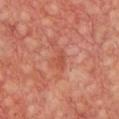The lesion was photographed on a routine skin check and not biopsied; there is no pathology result. The patient is a female aged 43 to 47. An algorithmic analysis of the crop reported an area of roughly 4.5 mm² and an outline eccentricity of about 0.75 (0 = round, 1 = elongated). It also reported a mean CIELAB color near L≈51 a*≈29 b*≈32, a lesion–skin lightness drop of about 6, and a lesion-to-skin contrast of about 5 (normalized; higher = more distinct). And it measured a border-irregularity rating of about 3/10 and a peripheral color-asymmetry measure near 0.5. Cropped from a total-body skin-imaging series; the visible field is about 15 mm. On the chest. Longest diameter approximately 3 mm.